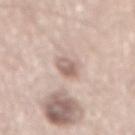Captured during whole-body skin photography for melanoma surveillance; the lesion was not biopsied.